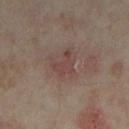workup: total-body-photography surveillance lesion; no biopsy
patient: female, aged around 35
imaging modality: 15 mm crop, total-body photography
diameter: ~3 mm (longest diameter)
automated lesion analysis: a footprint of about 4.5 mm², an outline eccentricity of about 0.7 (0 = round, 1 = elongated), and a shape-asymmetry score of about 0.65 (0 = symmetric); a lesion–skin lightness drop of about 6 and a normalized lesion–skin contrast near 5.5; a classifier nevus-likeness of about 0/100
anatomic site: the leg
lighting: cross-polarized illumination